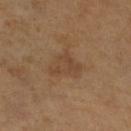Q: Was a biopsy performed?
A: total-body-photography surveillance lesion; no biopsy
Q: How was the tile lit?
A: cross-polarized
Q: Patient demographics?
A: female, in their mid- to late 50s
Q: What is the imaging modality?
A: total-body-photography crop, ~15 mm field of view
Q: Where on the body is the lesion?
A: the right lower leg
Q: Lesion size?
A: ≈3.5 mm